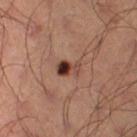Imaged during a routine full-body skin examination; the lesion was not biopsied and no histopathology is available.
The patient is a male roughly 50 years of age.
The total-body-photography lesion software estimated a footprint of about 5 mm², an outline eccentricity of about 0.8 (0 = round, 1 = elongated), and a symmetry-axis asymmetry near 0.2. The analysis additionally found an average lesion color of about L≈37 a*≈21 b*≈24 (CIELAB), a lesion–skin lightness drop of about 13, and a lesion-to-skin contrast of about 11 (normalized; higher = more distinct).
The lesion is on the right thigh.
The recorded lesion diameter is about 3 mm.
A region of skin cropped from a whole-body photographic capture, roughly 15 mm wide.